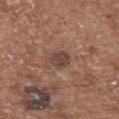Captured during whole-body skin photography for melanoma surveillance; the lesion was not biopsied.
A region of skin cropped from a whole-body photographic capture, roughly 15 mm wide.
The patient is a female aged approximately 75.
The lesion is located on the upper back.
The tile uses white-light illumination.